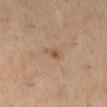• notes: imaged on a skin check; not biopsied
• lesion diameter: about 2.5 mm
• image: ~15 mm tile from a whole-body skin photo
• subject: female, aged approximately 50
• illumination: cross-polarized illumination
• location: the right lower leg